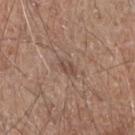This lesion was catalogued during total-body skin photography and was not selected for biopsy.
The tile uses white-light illumination.
Longest diameter approximately 2.5 mm.
The lesion is on the right forearm.
A male patient roughly 60 years of age.
A 15 mm crop from a total-body photograph taken for skin-cancer surveillance.